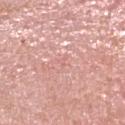Q: Is there a histopathology result?
A: no biopsy performed (imaged during a skin exam)
Q: What is the imaging modality?
A: ~15 mm crop, total-body skin-cancer survey
Q: What is the anatomic site?
A: the head or neck
Q: Patient demographics?
A: female, aged around 60
Q: What is the lesion's diameter?
A: about 1 mm
Q: How was the tile lit?
A: white-light illumination